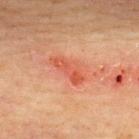{"biopsy_status": "not biopsied; imaged during a skin examination", "automated_metrics": {"cielab_L": 49, "cielab_a": 30, "cielab_b": 33, "vs_skin_darker_L": 8.0, "vs_skin_contrast_norm": 6.0}, "image": {"source": "total-body photography crop", "field_of_view_mm": 15}, "site": "upper back", "lesion_size": {"long_diameter_mm_approx": 4.5}, "lighting": "cross-polarized", "patient": {"sex": "female", "age_approx": 65}}